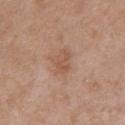Clinical impression:
No biopsy was performed on this lesion — it was imaged during a full skin examination and was not determined to be concerning.
Acquisition and patient details:
A 15 mm crop from a total-body photograph taken for skin-cancer surveillance. The lesion is on the chest. The patient is a female aged approximately 40.